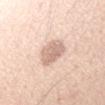The lesion was photographed on a routine skin check and not biopsied; there is no pathology result. Approximately 3.5 mm at its widest. The total-body-photography lesion software estimated a footprint of about 8.5 mm² and an outline eccentricity of about 0.55 (0 = round, 1 = elongated). The software also gave a mean CIELAB color near L≈70 a*≈18 b*≈27, about 12 CIELAB-L* units darker than the surrounding skin, and a lesion-to-skin contrast of about 7 (normalized; higher = more distinct). The software also gave border irregularity of about 2 on a 0–10 scale and a peripheral color-asymmetry measure near 1. And it measured a classifier nevus-likeness of about 5/100. The lesion is on the left forearm. This is a white-light tile. A 15 mm close-up tile from a total-body photography series done for melanoma screening. The subject is a female in their mid- to late 20s.